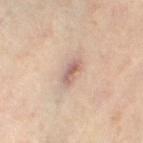biopsy_status: not biopsied; imaged during a skin examination
patient:
  sex: female
  age_approx: 50
automated_metrics:
  cielab_L: 59
  cielab_a: 18
  cielab_b: 24
  vs_skin_darker_L: 11.0
  vs_skin_contrast_norm: 7.5
  nevus_likeness_0_100: 0
  lesion_detection_confidence_0_100: 90
lighting: cross-polarized
image:
  source: total-body photography crop
  field_of_view_mm: 15
lesion_size:
  long_diameter_mm_approx: 3.0
site: right thigh